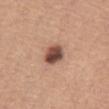Notes:
* notes · imaged on a skin check; not biopsied
* patient · female, approximately 55 years of age
* anatomic site · the abdomen
* illumination · white-light
* automated metrics · a border-irregularity index near 1.5/10 and radial color variation of about 2.5; an automated nevus-likeness rating near 90 out of 100
* lesion diameter · ≈3 mm
* image · total-body-photography crop, ~15 mm field of view